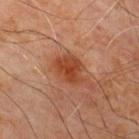| field | value |
|---|---|
| notes | catalogued during a skin exam; not biopsied |
| image | ~15 mm tile from a whole-body skin photo |
| body site | the chest |
| subject | male, approximately 70 years of age |